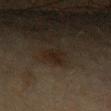follow-up: total-body-photography surveillance lesion; no biopsy
tile lighting: cross-polarized illumination
subject: male, aged approximately 70
site: the left upper arm
automated metrics: an average lesion color of about L≈15 a*≈9 b*≈17 (CIELAB), about 5 CIELAB-L* units darker than the surrounding skin, and a normalized lesion–skin contrast near 7.5; a classifier nevus-likeness of about 0/100 and lesion-presence confidence of about 100/100
lesion size: about 2.5 mm
imaging modality: total-body-photography crop, ~15 mm field of view